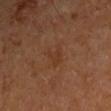Imaged during a routine full-body skin examination; the lesion was not biopsied and no histopathology is available. About 3 mm across. A 15 mm close-up tile from a total-body photography series done for melanoma screening. Located on the right upper arm. A male subject in their mid-60s.